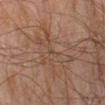Q: Was this lesion biopsied?
A: catalogued during a skin exam; not biopsied
Q: How was the tile lit?
A: cross-polarized illumination
Q: Lesion location?
A: the left lower leg
Q: How large is the lesion?
A: ≈6.5 mm
Q: Who is the patient?
A: male, aged approximately 45
Q: What is the imaging modality?
A: ~15 mm crop, total-body skin-cancer survey